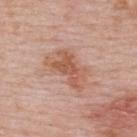| key | value |
|---|---|
| follow-up | total-body-photography surveillance lesion; no biopsy |
| lesion size | ~5.5 mm (longest diameter) |
| patient | female, in their 50s |
| illumination | white-light illumination |
| TBP lesion metrics | a shape eccentricity near 0.8 and two-axis asymmetry of about 0.3; an average lesion color of about L≈58 a*≈22 b*≈31 (CIELAB), a lesion–skin lightness drop of about 9, and a lesion-to-skin contrast of about 7 (normalized; higher = more distinct); an automated nevus-likeness rating near 20 out of 100 and a detector confidence of about 100 out of 100 that the crop contains a lesion |
| location | the back |
| imaging modality | total-body-photography crop, ~15 mm field of view |